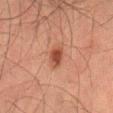Q: Is there a histopathology result?
A: total-body-photography surveillance lesion; no biopsy
Q: What is the lesion's diameter?
A: ≈3 mm
Q: Where on the body is the lesion?
A: the right thigh
Q: What is the imaging modality?
A: total-body-photography crop, ~15 mm field of view
Q: What lighting was used for the tile?
A: cross-polarized illumination
Q: What did automated image analysis measure?
A: an area of roughly 4 mm², a shape eccentricity near 0.85, and a shape-asymmetry score of about 0.3 (0 = symmetric); a mean CIELAB color near L≈37 a*≈22 b*≈26 and a lesion-to-skin contrast of about 9 (normalized; higher = more distinct); a classifier nevus-likeness of about 95/100
Q: Patient demographics?
A: male, aged 48–52